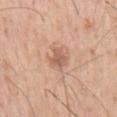Clinical impression: The lesion was photographed on a routine skin check and not biopsied; there is no pathology result. Image and clinical context: The subject is a male aged 53 to 57. Located on the right upper arm. A 15 mm crop from a total-body photograph taken for skin-cancer surveillance. The tile uses white-light illumination.